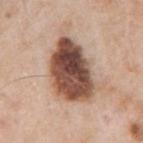  lighting: white-light
  lesion_size:
    long_diameter_mm_approx: 8.0
  image:
    source: total-body photography crop
    field_of_view_mm: 15
  patient:
    sex: male
    age_approx: 55
  site: left upper arm